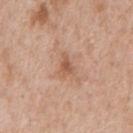Q: Is there a histopathology result?
A: imaged on a skin check; not biopsied
Q: Patient demographics?
A: male, about 65 years old
Q: What lighting was used for the tile?
A: white-light illumination
Q: What is the lesion's diameter?
A: about 3 mm
Q: Lesion location?
A: the back
Q: How was this image acquired?
A: 15 mm crop, total-body photography